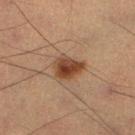The lesion was photographed on a routine skin check and not biopsied; there is no pathology result. From the left lower leg. A male subject roughly 55 years of age. This is a cross-polarized tile. Measured at roughly 3.5 mm in maximum diameter. A 15 mm close-up extracted from a 3D total-body photography capture.